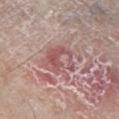{
  "biopsy_status": "not biopsied; imaged during a skin examination",
  "lighting": "white-light",
  "site": "left lower leg",
  "patient": {
    "sex": "male",
    "age_approx": 70
  },
  "image": {
    "source": "total-body photography crop",
    "field_of_view_mm": 15
  }
}